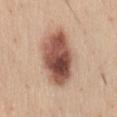notes: imaged on a skin check; not biopsied
TBP lesion metrics: a shape-asymmetry score of about 0.15 (0 = symmetric); an average lesion color of about L≈53 a*≈22 b*≈27 (CIELAB), roughly 19 lightness units darker than nearby skin, and a lesion-to-skin contrast of about 12 (normalized; higher = more distinct); lesion-presence confidence of about 100/100
image source: ~15 mm crop, total-body skin-cancer survey
subject: female, roughly 25 years of age
anatomic site: the front of the torso